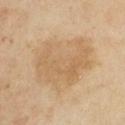Imaged during a routine full-body skin examination; the lesion was not biopsied and no histopathology is available. The lesion is located on the right upper arm. A male patient about 45 years old. About 8.5 mm across. A 15 mm close-up tile from a total-body photography series done for melanoma screening.